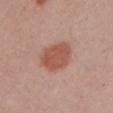workup=catalogued during a skin exam; not biopsied | location=the left upper arm | subject=male, in their 40s | image source=15 mm crop, total-body photography | lighting=white-light.